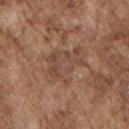Q: Is there a histopathology result?
A: catalogued during a skin exam; not biopsied
Q: Automated lesion metrics?
A: a lesion color around L≈47 a*≈19 b*≈27 in CIELAB and a lesion–skin lightness drop of about 6
Q: What are the patient's age and sex?
A: male, aged approximately 75
Q: Lesion size?
A: ~6 mm (longest diameter)
Q: How was the tile lit?
A: white-light illumination
Q: What is the imaging modality?
A: 15 mm crop, total-body photography
Q: Where on the body is the lesion?
A: the left upper arm